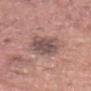<case>
  <biopsy_status>not biopsied; imaged during a skin examination</biopsy_status>
  <lighting>white-light</lighting>
  <site>head or neck</site>
  <image>
    <source>total-body photography crop</source>
    <field_of_view_mm>15</field_of_view_mm>
  </image>
  <lesion_size>
    <long_diameter_mm_approx>5.0</long_diameter_mm_approx>
  </lesion_size>
  <automated_metrics>
    <cielab_L>51</cielab_L>
    <cielab_a>17</cielab_a>
    <cielab_b>20</cielab_b>
    <vs_skin_darker_L>11.0</vs_skin_darker_L>
    <vs_skin_contrast_norm>8.5</vs_skin_contrast_norm>
    <border_irregularity_0_10>2.5</border_irregularity_0_10>
    <color_variation_0_10>4.5</color_variation_0_10>
    <peripheral_color_asymmetry>1.5</peripheral_color_asymmetry>
  </automated_metrics>
  <patient>
    <sex>male</sex>
    <age_approx>70</age_approx>
  </patient>
</case>